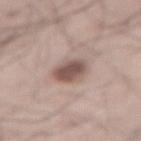biopsy_status: not biopsied; imaged during a skin examination
patient:
  sex: male
  age_approx: 65
automated_metrics:
  vs_skin_darker_L: 14.0
  vs_skin_contrast_norm: 9.5
  border_irregularity_0_10: 1.5
  color_variation_0_10: 3.5
  peripheral_color_asymmetry: 1.0
  lesion_detection_confidence_0_100: 100
lesion_size:
  long_diameter_mm_approx: 3.5
image:
  source: total-body photography crop
  field_of_view_mm: 15
site: lower back
lighting: white-light